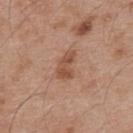Recorded during total-body skin imaging; not selected for excision or biopsy.
The lesion is located on the upper back.
Automated image analysis of the tile measured a lesion area of about 5.5 mm², an outline eccentricity of about 0.85 (0 = round, 1 = elongated), and two-axis asymmetry of about 0.45. The analysis additionally found a lesion color around L≈51 a*≈22 b*≈31 in CIELAB and a normalized border contrast of about 7. It also reported a nevus-likeness score of about 5/100 and a lesion-detection confidence of about 100/100.
The patient is a male aged approximately 55.
The tile uses white-light illumination.
A 15 mm crop from a total-body photograph taken for skin-cancer surveillance.